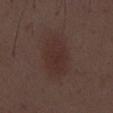notes = no biopsy performed (imaged during a skin exam); subject = male, aged 48–52; image source = ~15 mm tile from a whole-body skin photo; lesion diameter = ~6 mm (longest diameter).